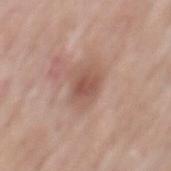Clinical impression:
Imaged during a routine full-body skin examination; the lesion was not biopsied and no histopathology is available.
Background:
From the mid back. The patient is a male about 55 years old. A 15 mm close-up tile from a total-body photography series done for melanoma screening.